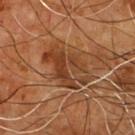biopsy status = no biopsy performed (imaged during a skin exam) | body site = the front of the torso | tile lighting = cross-polarized illumination | image = ~15 mm tile from a whole-body skin photo | TBP lesion metrics = a mean CIELAB color near L≈37 a*≈21 b*≈32, roughly 9 lightness units darker than nearby skin, and a normalized border contrast of about 7.5; a border-irregularity index near 4/10; a lesion-detection confidence of about 95/100 | patient = male, aged approximately 55 | lesion size = ≈6 mm.